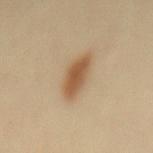Acquisition and patient details: A female subject approximately 40 years of age. Automated tile analysis of the lesion measured a shape eccentricity near 0.9. And it measured a lesion color around L≈56 a*≈18 b*≈35 in CIELAB, about 13 CIELAB-L* units darker than the surrounding skin, and a lesion-to-skin contrast of about 9 (normalized; higher = more distinct). Located on the mid back. A 15 mm close-up extracted from a 3D total-body photography capture. The lesion's longest dimension is about 5 mm. The tile uses cross-polarized illumination.